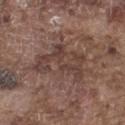Imaged during a routine full-body skin examination; the lesion was not biopsied and no histopathology is available.
A male patient, in their mid- to late 70s.
Imaged with white-light lighting.
Cropped from a total-body skin-imaging series; the visible field is about 15 mm.
Longest diameter approximately 6 mm.
Automated tile analysis of the lesion measured a lesion–skin lightness drop of about 8 and a lesion-to-skin contrast of about 6.5 (normalized; higher = more distinct). The analysis additionally found a border-irregularity rating of about 8.5/10 and a color-variation rating of about 4.5/10.
The lesion is on the left thigh.